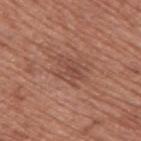Recorded during total-body skin imaging; not selected for excision or biopsy.
A male subject, about 75 years old.
From the right upper arm.
Imaged with white-light lighting.
Cropped from a whole-body photographic skin survey; the tile spans about 15 mm.